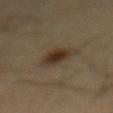biopsy_status: not biopsied; imaged during a skin examination
lesion_size:
  long_diameter_mm_approx: 3.5
site: mid back
patient:
  sex: male
  age_approx: 40
lighting: cross-polarized
image:
  source: total-body photography crop
  field_of_view_mm: 15